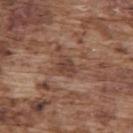diameter = ≈2.5 mm
illumination = white-light
patient = male, roughly 75 years of age
anatomic site = the upper back
acquisition = ~15 mm tile from a whole-body skin photo
TBP lesion metrics = a mean CIELAB color near L≈42 a*≈20 b*≈25, roughly 9 lightness units darker than nearby skin, and a normalized border contrast of about 7; a border-irregularity rating of about 3/10, a color-variation rating of about 2/10, and radial color variation of about 0.5